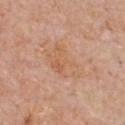notes — total-body-photography surveillance lesion; no biopsy
image — ~15 mm tile from a whole-body skin photo
anatomic site — the chest
size — ≈5 mm
lighting — white-light
automated metrics — an average lesion color of about L≈60 a*≈21 b*≈34 (CIELAB), roughly 5 lightness units darker than nearby skin, and a lesion-to-skin contrast of about 5 (normalized; higher = more distinct); a border-irregularity index near 3.5/10, a within-lesion color-variation index near 4/10, and radial color variation of about 1
patient — male, aged around 60